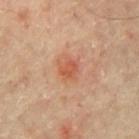Captured during whole-body skin photography for melanoma surveillance; the lesion was not biopsied. The lesion is on the mid back. The tile uses cross-polarized illumination. Approximately 2.5 mm at its widest. A male subject, aged 63–67. Cropped from a total-body skin-imaging series; the visible field is about 15 mm.